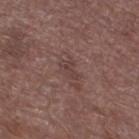Impression:
Recorded during total-body skin imaging; not selected for excision or biopsy.
Acquisition and patient details:
Imaged with white-light lighting. The lesion is on the leg. Longest diameter approximately 2.5 mm. The subject is a male in their mid-70s. The total-body-photography lesion software estimated an area of roughly 4 mm² and a shape eccentricity near 0.75. It also reported roughly 6 lightness units darker than nearby skin and a normalized lesion–skin contrast near 5. It also reported a detector confidence of about 95 out of 100 that the crop contains a lesion. Cropped from a total-body skin-imaging series; the visible field is about 15 mm.